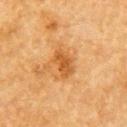• follow-up: total-body-photography surveillance lesion; no biopsy
• diameter: ~3.5 mm (longest diameter)
• patient: male, aged approximately 85 or older
• body site: the arm
• tile lighting: cross-polarized illumination
• automated lesion analysis: an area of roughly 5.5 mm², an eccentricity of roughly 0.85, and a symmetry-axis asymmetry near 0.3; border irregularity of about 3 on a 0–10 scale, internal color variation of about 2 on a 0–10 scale, and radial color variation of about 0.5; an automated nevus-likeness rating near 45 out of 100 and lesion-presence confidence of about 100/100
• image source: 15 mm crop, total-body photography